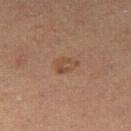Assessment:
No biopsy was performed on this lesion — it was imaged during a full skin examination and was not determined to be concerning.
Background:
A lesion tile, about 15 mm wide, cut from a 3D total-body photograph. About 3 mm across. Captured under cross-polarized illumination. The total-body-photography lesion software estimated a mean CIELAB color near L≈42 a*≈18 b*≈28, roughly 6 lightness units darker than nearby skin, and a normalized lesion–skin contrast near 6. The analysis additionally found border irregularity of about 2.5 on a 0–10 scale, a within-lesion color-variation index near 3/10, and peripheral color asymmetry of about 1. And it measured a classifier nevus-likeness of about 5/100 and lesion-presence confidence of about 100/100. The lesion is on the right thigh. The subject is a female about 30 years old.